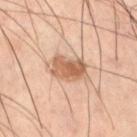Imaged during a routine full-body skin examination; the lesion was not biopsied and no histopathology is available.
The lesion is on the right thigh.
The patient is a male in their 60s.
The lesion's longest dimension is about 5 mm.
Imaged with cross-polarized lighting.
A 15 mm crop from a total-body photograph taken for skin-cancer surveillance.